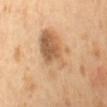Impression: Recorded during total-body skin imaging; not selected for excision or biopsy. Clinical summary: A female patient about 55 years old. Cropped from a whole-body photographic skin survey; the tile spans about 15 mm. The total-body-photography lesion software estimated an area of roughly 23 mm², an outline eccentricity of about 0.75 (0 = round, 1 = elongated), and two-axis asymmetry of about 0.3. The software also gave a mean CIELAB color near L≈64 a*≈19 b*≈37, about 10 CIELAB-L* units darker than the surrounding skin, and a normalized lesion–skin contrast near 6.5. The recorded lesion diameter is about 7 mm. Captured under cross-polarized illumination. The lesion is located on the mid back.